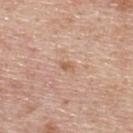  biopsy_status: not biopsied; imaged during a skin examination
  image:
    source: total-body photography crop
    field_of_view_mm: 15
  automated_metrics:
    cielab_L: 58
    cielab_a: 20
    cielab_b: 33
    vs_skin_darker_L: 9.0
  site: upper back
  patient:
    sex: male
    age_approx: 55
  lesion_size:
    long_diameter_mm_approx: 1.5
  lighting: white-light